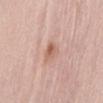workup: catalogued during a skin exam; not biopsied | anatomic site: the front of the torso | image-analysis metrics: a shape eccentricity near 0.8 and a shape-asymmetry score of about 0.3 (0 = symmetric); a border-irregularity rating of about 2.5/10 and peripheral color asymmetry of about 1.5 | subject: female, in their 30s | imaging modality: ~15 mm crop, total-body skin-cancer survey.